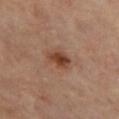notes=no biopsy performed (imaged during a skin exam)
diameter=~2.5 mm (longest diameter)
image=~15 mm crop, total-body skin-cancer survey
lighting=cross-polarized illumination
site=the left thigh
subject=female, roughly 50 years of age